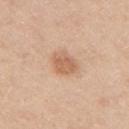Captured during whole-body skin photography for melanoma surveillance; the lesion was not biopsied. A roughly 15 mm field-of-view crop from a total-body skin photograph. Approximately 3 mm at its widest. Captured under white-light illumination. On the right upper arm. The total-body-photography lesion software estimated an average lesion color of about L≈62 a*≈21 b*≈34 (CIELAB), a lesion–skin lightness drop of about 10, and a lesion-to-skin contrast of about 6.5 (normalized; higher = more distinct). The analysis additionally found border irregularity of about 2 on a 0–10 scale, internal color variation of about 2 on a 0–10 scale, and peripheral color asymmetry of about 0.5. The patient is a female aged around 40.